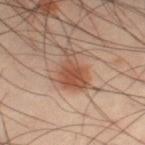Impression:
Imaged during a routine full-body skin examination; the lesion was not biopsied and no histopathology is available.
Context:
The lesion is on the leg. A male subject about 40 years old. This image is a 15 mm lesion crop taken from a total-body photograph. Automated tile analysis of the lesion measured a mean CIELAB color near L≈50 a*≈22 b*≈30, roughly 10 lightness units darker than nearby skin, and a lesion-to-skin contrast of about 8 (normalized; higher = more distinct). The lesion's longest dimension is about 4 mm.